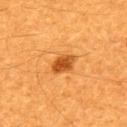Captured during whole-body skin photography for melanoma surveillance; the lesion was not biopsied. Located on the upper back. A male subject, roughly 60 years of age. A 15 mm close-up tile from a total-body photography series done for melanoma screening.